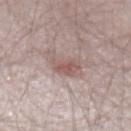Captured under white-light illumination. A 15 mm close-up extracted from a 3D total-body photography capture. A male patient, aged approximately 25. The lesion-visualizer software estimated a lesion area of about 7.5 mm², an eccentricity of roughly 0.8, and two-axis asymmetry of about 0.35. The software also gave an average lesion color of about L≈56 a*≈17 b*≈21 (CIELAB), roughly 9 lightness units darker than nearby skin, and a lesion-to-skin contrast of about 6.5 (normalized; higher = more distinct). And it measured a border-irregularity index near 4.5/10. It also reported an automated nevus-likeness rating near 15 out of 100 and a detector confidence of about 95 out of 100 that the crop contains a lesion. On the left forearm.